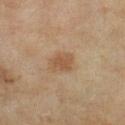Q: Was a biopsy performed?
A: imaged on a skin check; not biopsied
Q: How was this image acquired?
A: 15 mm crop, total-body photography
Q: Lesion location?
A: the left lower leg
Q: Who is the patient?
A: female, aged approximately 60